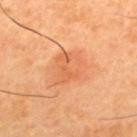biopsy_status: not biopsied; imaged during a skin examination
site: upper back
lesion_size:
  long_diameter_mm_approx: 4.5
image:
  source: total-body photography crop
  field_of_view_mm: 15
lighting: cross-polarized
patient:
  sex: male
  age_approx: 45